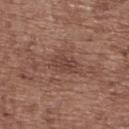tile lighting = white-light illumination
TBP lesion metrics = a border-irregularity index near 3/10 and a peripheral color-asymmetry measure near 1
diameter = ≈3.5 mm
patient = female, about 75 years old
imaging modality = 15 mm crop, total-body photography
anatomic site = the back